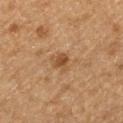follow-up=total-body-photography surveillance lesion; no biopsy
size=≈3 mm
patient=male, approximately 75 years of age
location=the leg
automated metrics=a border-irregularity rating of about 3.5/10, a within-lesion color-variation index near 2.5/10, and a peripheral color-asymmetry measure near 1; an automated nevus-likeness rating near 80 out of 100 and lesion-presence confidence of about 100/100
acquisition=15 mm crop, total-body photography